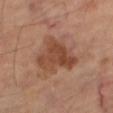{"patient": {"sex": "male", "age_approx": 70}, "site": "right thigh", "lesion_size": {"long_diameter_mm_approx": 5.0}, "automated_metrics": {"vs_skin_darker_L": 10.0, "vs_skin_contrast_norm": 8.0, "border_irregularity_0_10": 4.5, "color_variation_0_10": 4.0, "peripheral_color_asymmetry": 1.5, "nevus_likeness_0_100": 25}, "image": {"source": "total-body photography crop", "field_of_view_mm": 15}}